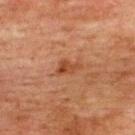Captured during whole-body skin photography for melanoma surveillance; the lesion was not biopsied. A male patient aged 73–77. The total-body-photography lesion software estimated about 8 CIELAB-L* units darker than the surrounding skin and a lesion-to-skin contrast of about 7 (normalized; higher = more distinct). The software also gave an automated nevus-likeness rating near 75 out of 100. Longest diameter approximately 3 mm. The lesion is located on the upper back. A 15 mm crop from a total-body photograph taken for skin-cancer surveillance. Imaged with cross-polarized lighting.